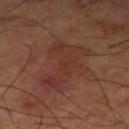biopsy_status: not biopsied; imaged during a skin examination
patient:
  sex: male
  age_approx: 65
image:
  source: total-body photography crop
  field_of_view_mm: 15
site: right thigh